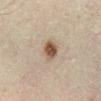tile lighting — cross-polarized illumination
patient — male, aged approximately 45
site — the leg
image — 15 mm crop, total-body photography
diameter — ~3 mm (longest diameter)
TBP lesion metrics — a lesion–skin lightness drop of about 12 and a lesion-to-skin contrast of about 10 (normalized; higher = more distinct)